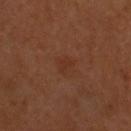The lesion was photographed on a routine skin check and not biopsied; there is no pathology result. Automated image analysis of the tile measured internal color variation of about 0.5 on a 0–10 scale and a peripheral color-asymmetry measure near 0.5. A male subject in their 60s. A roughly 15 mm field-of-view crop from a total-body skin photograph. The lesion is located on the head or neck.